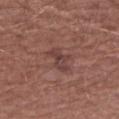  biopsy_status: not biopsied; imaged during a skin examination
  image:
    source: total-body photography crop
    field_of_view_mm: 15
  patient:
    sex: male
    age_approx: 65
  site: arm